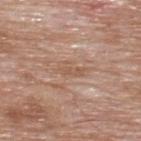| feature | finding |
|---|---|
| image-analysis metrics | an average lesion color of about L≈56 a*≈19 b*≈30 (CIELAB), a lesion–skin lightness drop of about 7, and a normalized lesion–skin contrast near 5.5; a nevus-likeness score of about 0/100 |
| subject | male, aged approximately 60 |
| body site | the back |
| size | ~3.5 mm (longest diameter) |
| lighting | white-light |
| acquisition | ~15 mm tile from a whole-body skin photo |